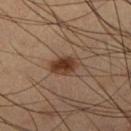lighting — cross-polarized
TBP lesion metrics — a lesion area of about 7 mm², an eccentricity of roughly 0.75, and a shape-asymmetry score of about 0.25 (0 = symmetric); a lesion–skin lightness drop of about 12 and a lesion-to-skin contrast of about 10.5 (normalized; higher = more distinct); a nevus-likeness score of about 95/100 and lesion-presence confidence of about 100/100
image — total-body-photography crop, ~15 mm field of view
location — the right lower leg
subject — male, about 65 years old
lesion size — ≈3.5 mm